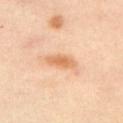image-analysis metrics = a border-irregularity rating of about 2/10, a color-variation rating of about 2.5/10, and peripheral color asymmetry of about 0.5; a classifier nevus-likeness of about 90/100 and lesion-presence confidence of about 100/100
image source = total-body-photography crop, ~15 mm field of view
patient = female, in their 40s
location = the left upper arm